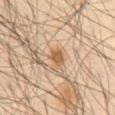Impression:
Imaged during a routine full-body skin examination; the lesion was not biopsied and no histopathology is available.
Acquisition and patient details:
The recorded lesion diameter is about 2.5 mm. From the abdomen. Imaged with cross-polarized lighting. The subject is a male about 65 years old. A region of skin cropped from a whole-body photographic capture, roughly 15 mm wide.